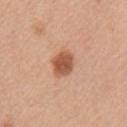Q: Was a biopsy performed?
A: imaged on a skin check; not biopsied
Q: Lesion size?
A: about 3 mm
Q: What did automated image analysis measure?
A: an area of roughly 7 mm² and an eccentricity of roughly 0.5; an average lesion color of about L≈55 a*≈25 b*≈33 (CIELAB), a lesion–skin lightness drop of about 14, and a normalized lesion–skin contrast near 9; border irregularity of about 1.5 on a 0–10 scale, a color-variation rating of about 3/10, and peripheral color asymmetry of about 1
Q: Who is the patient?
A: female, in their 40s
Q: How was the tile lit?
A: white-light illumination
Q: How was this image acquired?
A: ~15 mm tile from a whole-body skin photo
Q: Where on the body is the lesion?
A: the left upper arm